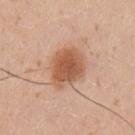No biopsy was performed on this lesion — it was imaged during a full skin examination and was not determined to be concerning.
Measured at roughly 4.5 mm in maximum diameter.
The total-body-photography lesion software estimated a border-irregularity index near 1.5/10, internal color variation of about 4 on a 0–10 scale, and peripheral color asymmetry of about 1. It also reported an automated nevus-likeness rating near 100 out of 100.
A male subject aged 33–37.
This is a white-light tile.
A 15 mm crop from a total-body photograph taken for skin-cancer surveillance.
Located on the left upper arm.